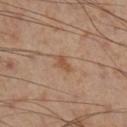Findings:
– notes · catalogued during a skin exam; not biopsied
– subject · male, aged 53–57
– diameter · ~2.5 mm (longest diameter)
– anatomic site · the right lower leg
– image source · ~15 mm tile from a whole-body skin photo
– illumination · cross-polarized illumination